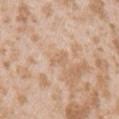| field | value |
|---|---|
| follow-up | imaged on a skin check; not biopsied |
| anatomic site | the left upper arm |
| automated metrics | an area of roughly 3 mm², an eccentricity of roughly 0.8, and a shape-asymmetry score of about 0.4 (0 = symmetric) |
| patient | female, in their mid-20s |
| image source | total-body-photography crop, ~15 mm field of view |
| tile lighting | white-light illumination |